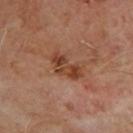Assessment:
Part of a total-body skin-imaging series; this lesion was reviewed on a skin check and was not flagged for biopsy.
Acquisition and patient details:
A close-up tile cropped from a whole-body skin photograph, about 15 mm across. The lesion is located on the upper back. A male patient, roughly 65 years of age.